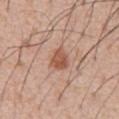The lesion was tiled from a total-body skin photograph and was not biopsied. A male subject aged around 55. Cropped from a total-body skin-imaging series; the visible field is about 15 mm. The lesion is located on the abdomen. Captured under white-light illumination. Automated image analysis of the tile measured an area of roughly 5 mm², an outline eccentricity of about 0.6 (0 = round, 1 = elongated), and a symmetry-axis asymmetry near 0.25. The software also gave an average lesion color of about L≈54 a*≈23 b*≈31 (CIELAB), a lesion–skin lightness drop of about 11, and a lesion-to-skin contrast of about 8 (normalized; higher = more distinct).